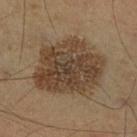Recorded during total-body skin imaging; not selected for excision or biopsy.
This image is a 15 mm lesion crop taken from a total-body photograph.
A male subject roughly 55 years of age.
An algorithmic analysis of the crop reported a lesion area of about 37 mm², a shape eccentricity near 0.6, and a shape-asymmetry score of about 0.2 (0 = symmetric). The software also gave a lesion color around L≈41 a*≈14 b*≈28 in CIELAB, about 11 CIELAB-L* units darker than the surrounding skin, and a normalized lesion–skin contrast near 9.5. The analysis additionally found an automated nevus-likeness rating near 25 out of 100 and lesion-presence confidence of about 100/100.
From the right lower leg.
Longest diameter approximately 8 mm.